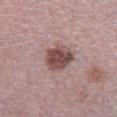Clinical impression:
Captured during whole-body skin photography for melanoma surveillance; the lesion was not biopsied.
Clinical summary:
The patient is a male in their 50s. The tile uses white-light illumination. A 15 mm crop from a total-body photograph taken for skin-cancer surveillance. The total-body-photography lesion software estimated border irregularity of about 2 on a 0–10 scale, a within-lesion color-variation index near 4/10, and a peripheral color-asymmetry measure near 1.5. The software also gave a classifier nevus-likeness of about 45/100. The lesion is on the left lower leg.